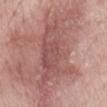This lesion was catalogued during total-body skin photography and was not selected for biopsy. The lesion is on the mid back. Captured under white-light illumination. A close-up tile cropped from a whole-body skin photograph, about 15 mm across. Automated tile analysis of the lesion measured an eccentricity of roughly 0.9 and a symmetry-axis asymmetry near 0.45. It also reported roughly 12 lightness units darker than nearby skin. A male patient, approximately 65 years of age. The recorded lesion diameter is about 13 mm.